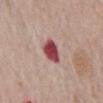This lesion was catalogued during total-body skin photography and was not selected for biopsy.
The tile uses white-light illumination.
A male subject, approximately 75 years of age.
A lesion tile, about 15 mm wide, cut from a 3D total-body photograph.
From the abdomen.
Measured at roughly 3 mm in maximum diameter.